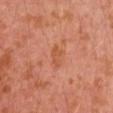Q: Is there a histopathology result?
A: catalogued during a skin exam; not biopsied
Q: Where on the body is the lesion?
A: the left arm
Q: How was this image acquired?
A: ~15 mm crop, total-body skin-cancer survey
Q: Lesion size?
A: about 3 mm
Q: What are the patient's age and sex?
A: male, aged 28–32